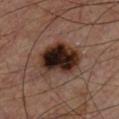Acquisition and patient details: The lesion-visualizer software estimated an area of roughly 18 mm², a shape eccentricity near 0.65, and two-axis asymmetry of about 0.2. The analysis additionally found a border-irregularity index near 2.5/10, a within-lesion color-variation index near 10/10, and a peripheral color-asymmetry measure near 3.5. On the chest. The patient is a male about 55 years old. This image is a 15 mm lesion crop taken from a total-body photograph. Imaged with cross-polarized lighting.